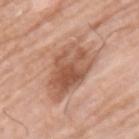Captured during whole-body skin photography for melanoma surveillance; the lesion was not biopsied.
The recorded lesion diameter is about 7 mm.
Located on the back.
The total-body-photography lesion software estimated a normalized border contrast of about 8. The software also gave an automated nevus-likeness rating near 65 out of 100 and a lesion-detection confidence of about 100/100.
This is a white-light tile.
A 15 mm crop from a total-body photograph taken for skin-cancer surveillance.
A male subject in their mid-60s.